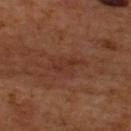Assessment: Part of a total-body skin-imaging series; this lesion was reviewed on a skin check and was not flagged for biopsy. Background: The lesion is on the upper back. Imaged with cross-polarized lighting. A male subject aged 68 to 72. Longest diameter approximately 4 mm. A lesion tile, about 15 mm wide, cut from a 3D total-body photograph.